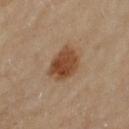image source: total-body-photography crop, ~15 mm field of view
site: the left upper arm
patient: female, aged 58 to 62
size: ≈4.5 mm